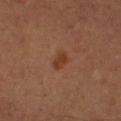No biopsy was performed on this lesion — it was imaged during a full skin examination and was not determined to be concerning.
A male patient, aged 63 to 67.
This is a cross-polarized tile.
The lesion-visualizer software estimated an eccentricity of roughly 0.75. The software also gave an average lesion color of about L≈32 a*≈20 b*≈28 (CIELAB) and a normalized lesion–skin contrast near 7.5. The analysis additionally found a border-irregularity index near 2.5/10 and radial color variation of about 1.
This image is a 15 mm lesion crop taken from a total-body photograph.
On the right upper arm.